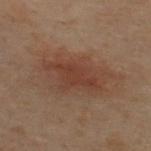{"biopsy_status": "not biopsied; imaged during a skin examination", "lighting": "cross-polarized", "patient": {"sex": "male", "age_approx": 50}, "automated_metrics": {"area_mm2_approx": 22.0, "eccentricity": 0.8, "border_irregularity_0_10": 3.5, "color_variation_0_10": 3.5, "peripheral_color_asymmetry": 1.0, "nevus_likeness_0_100": 10, "lesion_detection_confidence_0_100": 100}, "site": "upper back", "image": {"source": "total-body photography crop", "field_of_view_mm": 15}, "lesion_size": {"long_diameter_mm_approx": 7.5}}